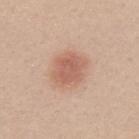Part of a total-body skin-imaging series; this lesion was reviewed on a skin check and was not flagged for biopsy.
A 15 mm close-up extracted from a 3D total-body photography capture.
A female subject, approximately 25 years of age.
The recorded lesion diameter is about 4 mm.
On the left upper arm.
The tile uses white-light illumination.